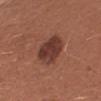Recorded during total-body skin imaging; not selected for excision or biopsy.
A female patient about 25 years old.
Imaged with white-light lighting.
The recorded lesion diameter is about 4.5 mm.
A 15 mm close-up extracted from a 3D total-body photography capture.
From the arm.